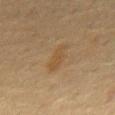The lesion was tiled from a total-body skin photograph and was not biopsied. Approximately 4 mm at its widest. The patient is a male about 70 years old. A region of skin cropped from a whole-body photographic capture, roughly 15 mm wide. Located on the chest. Automated tile analysis of the lesion measured a lesion color around L≈40 a*≈13 b*≈30 in CIELAB, roughly 5 lightness units darker than nearby skin, and a normalized border contrast of about 5.5. The analysis additionally found a classifier nevus-likeness of about 0/100 and a lesion-detection confidence of about 100/100.